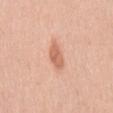Assessment:
This lesion was catalogued during total-body skin photography and was not selected for biopsy.
Acquisition and patient details:
Captured under white-light illumination. A male subject, approximately 40 years of age. About 4 mm across. The lesion-visualizer software estimated border irregularity of about 2.5 on a 0–10 scale. The analysis additionally found a nevus-likeness score of about 85/100 and a lesion-detection confidence of about 100/100. From the mid back. Cropped from a whole-body photographic skin survey; the tile spans about 15 mm.